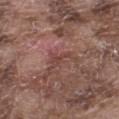Impression:
No biopsy was performed on this lesion — it was imaged during a full skin examination and was not determined to be concerning.
Background:
A roughly 15 mm field-of-view crop from a total-body skin photograph. A male patient, in their mid- to late 70s. The tile uses white-light illumination. About 3 mm across. Located on the left thigh. The total-body-photography lesion software estimated an average lesion color of about L≈43 a*≈22 b*≈23 (CIELAB), about 7 CIELAB-L* units darker than the surrounding skin, and a lesion-to-skin contrast of about 5.5 (normalized; higher = more distinct). It also reported a border-irregularity index near 6/10.